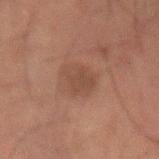Captured during whole-body skin photography for melanoma surveillance; the lesion was not biopsied.
Longest diameter approximately 4 mm.
Cropped from a total-body skin-imaging series; the visible field is about 15 mm.
The tile uses cross-polarized illumination.
The lesion is on the left forearm.
The patient is a male aged approximately 50.